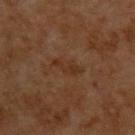No biopsy was performed on this lesion — it was imaged during a full skin examination and was not determined to be concerning.